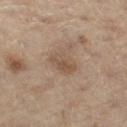Recorded during total-body skin imaging; not selected for excision or biopsy.
Measured at roughly 3 mm in maximum diameter.
Located on the left thigh.
The lesion-visualizer software estimated an area of roughly 6 mm², an eccentricity of roughly 0.75, and a shape-asymmetry score of about 0.2 (0 = symmetric). And it measured an average lesion color of about L≈52 a*≈16 b*≈29 (CIELAB) and about 8 CIELAB-L* units darker than the surrounding skin.
A 15 mm crop from a total-body photograph taken for skin-cancer surveillance.
A male subject roughly 70 years of age.